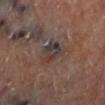Q: Was this lesion biopsied?
A: imaged on a skin check; not biopsied
Q: What lighting was used for the tile?
A: cross-polarized illumination
Q: Automated lesion metrics?
A: an area of roughly 8.5 mm² and an eccentricity of roughly 0.75; a border-irregularity index near 3/10 and internal color variation of about 10 on a 0–10 scale; a classifier nevus-likeness of about 0/100 and a lesion-detection confidence of about 80/100
Q: How large is the lesion?
A: about 4 mm
Q: What kind of image is this?
A: ~15 mm crop, total-body skin-cancer survey
Q: Lesion location?
A: the leg